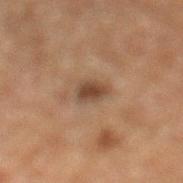follow-up: no biopsy performed (imaged during a skin exam); acquisition: ~15 mm tile from a whole-body skin photo; patient: male, aged approximately 75; location: the left lower leg.